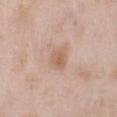Q: Is there a histopathology result?
A: imaged on a skin check; not biopsied
Q: Patient demographics?
A: male, approximately 55 years of age
Q: Lesion location?
A: the abdomen
Q: What kind of image is this?
A: 15 mm crop, total-body photography